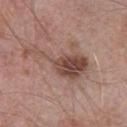Assessment:
Imaged during a routine full-body skin examination; the lesion was not biopsied and no histopathology is available.
Clinical summary:
Located on the front of the torso. A lesion tile, about 15 mm wide, cut from a 3D total-body photograph. A male subject in their mid- to late 70s. Measured at roughly 8 mm in maximum diameter.